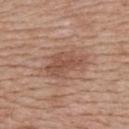Imaged during a routine full-body skin examination; the lesion was not biopsied and no histopathology is available.
A close-up tile cropped from a whole-body skin photograph, about 15 mm across.
The tile uses white-light illumination.
From the upper back.
The lesion's longest dimension is about 5 mm.
An algorithmic analysis of the crop reported an eccentricity of roughly 0.8. The analysis additionally found an automated nevus-likeness rating near 50 out of 100 and a lesion-detection confidence of about 100/100.
A female subject about 50 years old.